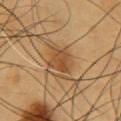Captured during whole-body skin photography for melanoma surveillance; the lesion was not biopsied.
The patient is a male in their 60s.
An algorithmic analysis of the crop reported an outline eccentricity of about 0.4 (0 = round, 1 = elongated) and a shape-asymmetry score of about 0.25 (0 = symmetric). And it measured an average lesion color of about L≈48 a*≈20 b*≈37 (CIELAB), roughly 9 lightness units darker than nearby skin, and a normalized lesion–skin contrast near 6.5. And it measured an automated nevus-likeness rating near 75 out of 100.
On the chest.
Imaged with cross-polarized lighting.
A region of skin cropped from a whole-body photographic capture, roughly 15 mm wide.
Measured at roughly 3 mm in maximum diameter.